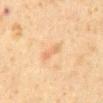Case summary:
- body site: the chest
- imaging modality: 15 mm crop, total-body photography
- patient: male, roughly 60 years of age